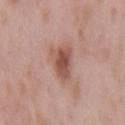Captured during whole-body skin photography for melanoma surveillance; the lesion was not biopsied. The lesion-visualizer software estimated border irregularity of about 3.5 on a 0–10 scale, a color-variation rating of about 4/10, and radial color variation of about 1. The patient is a male aged approximately 55. The lesion is on the back. A 15 mm close-up extracted from a 3D total-body photography capture. Captured under white-light illumination. About 4 mm across.